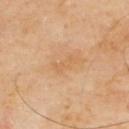follow-up: catalogued during a skin exam; not biopsied
automated lesion analysis: a footprint of about 3 mm², an outline eccentricity of about 0.9 (0 = round, 1 = elongated), and a shape-asymmetry score of about 0.45 (0 = symmetric); border irregularity of about 5.5 on a 0–10 scale and a color-variation rating of about 0/10; an automated nevus-likeness rating near 0 out of 100 and lesion-presence confidence of about 100/100
image source: ~15 mm tile from a whole-body skin photo
subject: male, in their mid- to late 60s
diameter: ~3 mm (longest diameter)
tile lighting: cross-polarized illumination
body site: the upper back